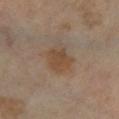  biopsy_status: not biopsied; imaged during a skin examination
  image:
    source: total-body photography crop
    field_of_view_mm: 15
  site: left lower leg
  patient:
    sex: male
    age_approx: 65